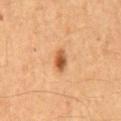Notes:
• follow-up: imaged on a skin check; not biopsied
• image-analysis metrics: a lesion color around L≈46 a*≈22 b*≈34 in CIELAB; a border-irregularity index near 2.5/10 and a color-variation rating of about 4/10; a lesion-detection confidence of about 100/100
• subject: male, aged around 65
• size: about 3 mm
• body site: the mid back
• acquisition: 15 mm crop, total-body photography
• illumination: cross-polarized